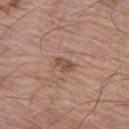No biopsy was performed on this lesion — it was imaged during a full skin examination and was not determined to be concerning.
This image is a 15 mm lesion crop taken from a total-body photograph.
Captured under white-light illumination.
The lesion is located on the left thigh.
A male subject, approximately 65 years of age.
The lesion's longest dimension is about 2.5 mm.